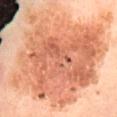workup: no biopsy performed (imaged during a skin exam) | subject: female, aged 53–57 | tile lighting: cross-polarized | location: the abdomen | lesion diameter: ~12 mm (longest diameter) | image source: ~15 mm tile from a whole-body skin photo.